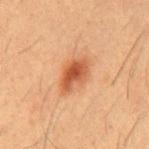<lesion>
<biopsy_status>not biopsied; imaged during a skin examination</biopsy_status>
<image>
  <source>total-body photography crop</source>
  <field_of_view_mm>15</field_of_view_mm>
</image>
<lesion_size>
  <long_diameter_mm_approx>4.5</long_diameter_mm_approx>
</lesion_size>
<automated_metrics>
  <area_mm2_approx>8.5</area_mm2_approx>
  <eccentricity>0.8</eccentricity>
  <shape_asymmetry>0.25</shape_asymmetry>
  <nevus_likeness_0_100>95</nevus_likeness_0_100>
  <lesion_detection_confidence_0_100>100</lesion_detection_confidence_0_100>
</automated_metrics>
<patient>
  <sex>male</sex>
  <age_approx>55</age_approx>
</patient>
<site>chest</site>
</lesion>